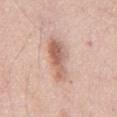{
  "biopsy_status": "not biopsied; imaged during a skin examination",
  "site": "chest",
  "image": {
    "source": "total-body photography crop",
    "field_of_view_mm": 15
  },
  "patient": {
    "sex": "male",
    "age_approx": 55
  },
  "automated_metrics": {
    "area_mm2_approx": 12.0,
    "eccentricity": 0.95,
    "shape_asymmetry": 0.25,
    "vs_skin_darker_L": 12.0,
    "vs_skin_contrast_norm": 7.5,
    "color_variation_0_10": 6.0,
    "peripheral_color_asymmetry": 2.0,
    "nevus_likeness_0_100": 100,
    "lesion_detection_confidence_0_100": 100
  }
}